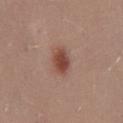{
  "biopsy_status": "not biopsied; imaged during a skin examination",
  "site": "mid back",
  "lesion_size": {
    "long_diameter_mm_approx": 3.5
  },
  "lighting": "white-light",
  "patient": {
    "sex": "female",
    "age_approx": 25
  },
  "image": {
    "source": "total-body photography crop",
    "field_of_view_mm": 15
  }
}